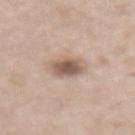Background:
On the abdomen. A female patient, approximately 75 years of age. Cropped from a total-body skin-imaging series; the visible field is about 15 mm.